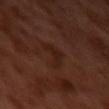Part of a total-body skin-imaging series; this lesion was reviewed on a skin check and was not flagged for biopsy. The recorded lesion diameter is about 3.5 mm. A male subject, about 30 years old. The lesion is on the left upper arm. A roughly 15 mm field-of-view crop from a total-body skin photograph.